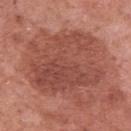The lesion was tiled from a total-body skin photograph and was not biopsied. A roughly 15 mm field-of-view crop from a total-body skin photograph. Captured under white-light illumination. The lesion is on the left upper arm. Automated tile analysis of the lesion measured a lesion–skin lightness drop of about 9 and a normalized lesion–skin contrast near 6.5. It also reported a border-irregularity rating of about 5/10, a color-variation rating of about 5/10, and peripheral color asymmetry of about 2. A female subject, aged 48–52.